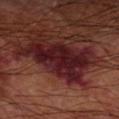No biopsy was performed on this lesion — it was imaged during a full skin examination and was not determined to be concerning.
Captured under cross-polarized illumination.
This image is a 15 mm lesion crop taken from a total-body photograph.
The recorded lesion diameter is about 8 mm.
A male patient in their 60s.
Located on the arm.
An algorithmic analysis of the crop reported an area of roughly 22 mm², an eccentricity of roughly 0.85, and two-axis asymmetry of about 0.4. And it measured a mean CIELAB color near L≈20 a*≈25 b*≈17 and a normalized lesion–skin contrast near 14.5. And it measured a border-irregularity rating of about 6/10, internal color variation of about 3.5 on a 0–10 scale, and radial color variation of about 1.